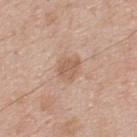Impression:
The lesion was tiled from a total-body skin photograph and was not biopsied.